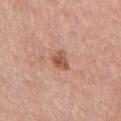Imaged during a routine full-body skin examination; the lesion was not biopsied and no histopathology is available.
This image is a 15 mm lesion crop taken from a total-body photograph.
A male subject, roughly 60 years of age.
The lesion is located on the chest.